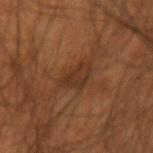Impression:
The lesion was tiled from a total-body skin photograph and was not biopsied.
Background:
Imaged with cross-polarized lighting. A 15 mm close-up extracted from a 3D total-body photography capture. Measured at roughly 4.5 mm in maximum diameter. Located on the right forearm. The patient is a male in their mid- to late 50s.